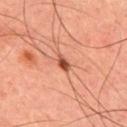{
  "biopsy_status": "not biopsied; imaged during a skin examination",
  "lighting": "cross-polarized",
  "patient": {
    "sex": "male",
    "age_approx": 45
  },
  "site": "upper back",
  "image": {
    "source": "total-body photography crop",
    "field_of_view_mm": 15
  },
  "lesion_size": {
    "long_diameter_mm_approx": 2.0
  },
  "automated_metrics": {
    "area_mm2_approx": 2.5,
    "shape_asymmetry": 0.3,
    "cielab_L": 48,
    "cielab_a": 29,
    "cielab_b": 33,
    "vs_skin_contrast_norm": 10.0,
    "color_variation_0_10": 0.5,
    "peripheral_color_asymmetry": 0.0,
    "nevus_likeness_0_100": 95,
    "lesion_detection_confidence_0_100": 100
  }
}